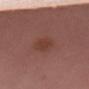- biopsy status · total-body-photography surveillance lesion; no biopsy
- lighting · white-light
- imaging modality · ~15 mm crop, total-body skin-cancer survey
- patient · male, aged approximately 40
- site · the right upper arm
- automated lesion analysis · a footprint of about 4 mm² and a shape-asymmetry score of about 0.2 (0 = symmetric); a border-irregularity index near 2/10, a within-lesion color-variation index near 1.5/10, and radial color variation of about 0.5; an automated nevus-likeness rating near 15 out of 100 and lesion-presence confidence of about 100/100